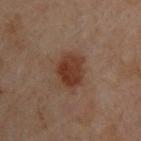Assessment: Captured during whole-body skin photography for melanoma surveillance; the lesion was not biopsied. Image and clinical context: From the upper back. Captured under cross-polarized illumination. A lesion tile, about 15 mm wide, cut from a 3D total-body photograph. About 4 mm across. The patient is a male aged 48 to 52.